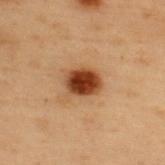biopsy status: total-body-photography surveillance lesion; no biopsy
patient: male, about 55 years old
lesion size: ≈3.5 mm
TBP lesion metrics: a footprint of about 8 mm², an outline eccentricity of about 0.65 (0 = round, 1 = elongated), and a symmetry-axis asymmetry near 0.15; a mean CIELAB color near L≈34 a*≈22 b*≈31 and roughly 16 lightness units darker than nearby skin; a border-irregularity rating of about 1.5/10, a within-lesion color-variation index near 4.5/10, and radial color variation of about 1; a nevus-likeness score of about 100/100 and a lesion-detection confidence of about 100/100
imaging modality: total-body-photography crop, ~15 mm field of view
location: the upper back
lighting: cross-polarized